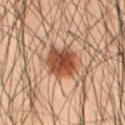{
  "patient": {
    "sex": "male",
    "age_approx": 55
  },
  "image": {
    "source": "total-body photography crop",
    "field_of_view_mm": 15
  },
  "lesion_size": {
    "long_diameter_mm_approx": 4.0
  },
  "site": "abdomen"
}